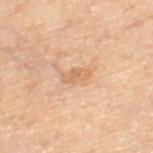Recorded during total-body skin imaging; not selected for excision or biopsy. A male subject aged around 70. A 15 mm close-up tile from a total-body photography series done for melanoma screening. The lesion is located on the left thigh.